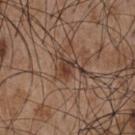This lesion was catalogued during total-body skin photography and was not selected for biopsy. This image is a 15 mm lesion crop taken from a total-body photograph. Automated image analysis of the tile measured an area of roughly 6 mm² and a symmetry-axis asymmetry near 0.65. A male patient, aged 53 to 57. The tile uses white-light illumination. Located on the front of the torso.